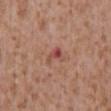| feature | finding |
|---|---|
| notes | total-body-photography surveillance lesion; no biopsy |
| subject | male, roughly 45 years of age |
| image source | ~15 mm crop, total-body skin-cancer survey |
| anatomic site | the chest |